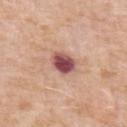Acquisition and patient details: Automated tile analysis of the lesion measured an area of roughly 6.5 mm² and an eccentricity of roughly 0.55. The software also gave an average lesion color of about L≈50 a*≈26 b*≈22 (CIELAB), a lesion–skin lightness drop of about 18, and a normalized lesion–skin contrast near 13. The software also gave a border-irregularity rating of about 1.5/10 and peripheral color asymmetry of about 1.5. The software also gave an automated nevus-likeness rating near 20 out of 100 and a lesion-detection confidence of about 100/100. Cropped from a whole-body photographic skin survey; the tile spans about 15 mm. From the left upper arm. Captured under white-light illumination. A female patient aged 58 to 62. Measured at roughly 3 mm in maximum diameter.